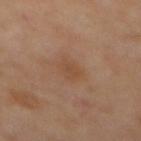Assessment:
The lesion was photographed on a routine skin check and not biopsied; there is no pathology result.
Context:
The subject is a female in their 50s. A 15 mm crop from a total-body photograph taken for skin-cancer surveillance. Measured at roughly 3 mm in maximum diameter. From the mid back. Imaged with cross-polarized lighting. The total-body-photography lesion software estimated an area of roughly 4.5 mm², an outline eccentricity of about 0.8 (0 = round, 1 = elongated), and two-axis asymmetry of about 0.25.